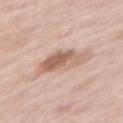Impression: This lesion was catalogued during total-body skin photography and was not selected for biopsy. Image and clinical context: Captured under white-light illumination. Cropped from a whole-body photographic skin survey; the tile spans about 15 mm. Longest diameter approximately 5 mm. The subject is a female about 50 years old. The lesion is located on the left upper arm.